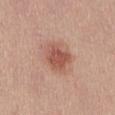Q: Was this lesion biopsied?
A: no biopsy performed (imaged during a skin exam)
Q: What kind of image is this?
A: ~15 mm tile from a whole-body skin photo
Q: Who is the patient?
A: female, roughly 45 years of age
Q: What lighting was used for the tile?
A: white-light
Q: How large is the lesion?
A: about 3.5 mm
Q: Lesion location?
A: the abdomen